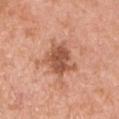Q: Was this lesion biopsied?
A: total-body-photography surveillance lesion; no biopsy
Q: Who is the patient?
A: male, about 70 years old
Q: Automated lesion metrics?
A: an area of roughly 11 mm², a shape eccentricity near 0.55, and a shape-asymmetry score of about 0.3 (0 = symmetric); roughly 13 lightness units darker than nearby skin and a lesion-to-skin contrast of about 8.5 (normalized; higher = more distinct); a border-irregularity index near 4/10, a within-lesion color-variation index near 3.5/10, and a peripheral color-asymmetry measure near 1; an automated nevus-likeness rating near 5 out of 100 and lesion-presence confidence of about 100/100
Q: How was this image acquired?
A: ~15 mm crop, total-body skin-cancer survey
Q: What is the lesion's diameter?
A: ~4 mm (longest diameter)
Q: Lesion location?
A: the right upper arm